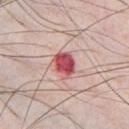Part of a total-body skin-imaging series; this lesion was reviewed on a skin check and was not flagged for biopsy. Located on the chest. About 3.5 mm across. A male subject aged 33–37. This image is a 15 mm lesion crop taken from a total-body photograph. This is a white-light tile. Automated tile analysis of the lesion measured an outline eccentricity of about 0.6 (0 = round, 1 = elongated). It also reported a lesion color around L≈54 a*≈34 b*≈23 in CIELAB. The software also gave border irregularity of about 2.5 on a 0–10 scale, a color-variation rating of about 5/10, and peripheral color asymmetry of about 1.5.